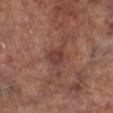Part of a total-body skin-imaging series; this lesion was reviewed on a skin check and was not flagged for biopsy.
About 2.5 mm across.
The total-body-photography lesion software estimated a border-irregularity index near 2.5/10 and peripheral color asymmetry of about 1.
This is a cross-polarized tile.
The patient is a male about 65 years old.
On the left lower leg.
A region of skin cropped from a whole-body photographic capture, roughly 15 mm wide.